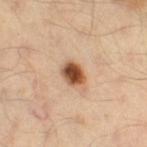| field | value |
|---|---|
| notes | no biopsy performed (imaged during a skin exam) |
| location | the right thigh |
| size | ~3 mm (longest diameter) |
| subject | male, aged 38–42 |
| image source | 15 mm crop, total-body photography |